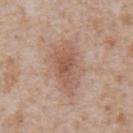Clinical impression: Recorded during total-body skin imaging; not selected for excision or biopsy. Image and clinical context: A male subject, aged approximately 65. The lesion is located on the abdomen. A roughly 15 mm field-of-view crop from a total-body skin photograph.